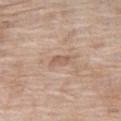No biopsy was performed on this lesion — it was imaged during a full skin examination and was not determined to be concerning. Imaged with white-light lighting. The patient is a female aged around 75. A roughly 15 mm field-of-view crop from a total-body skin photograph. From the right thigh. Approximately 2.5 mm at its widest.